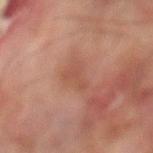The tile uses cross-polarized illumination. From the leg. The lesion's longest dimension is about 4 mm. The subject is a male approximately 75 years of age. The lesion-visualizer software estimated an area of roughly 4.5 mm² and an outline eccentricity of about 0.9 (0 = round, 1 = elongated). The analysis additionally found about 6 CIELAB-L* units darker than the surrounding skin. The software also gave a classifier nevus-likeness of about 0/100 and a lesion-detection confidence of about 95/100. A 15 mm close-up extracted from a 3D total-body photography capture.